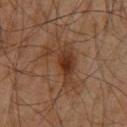Impression:
This lesion was catalogued during total-body skin photography and was not selected for biopsy.
Background:
From the chest. A 15 mm close-up extracted from a 3D total-body photography capture. A male subject, aged approximately 60. Automated tile analysis of the lesion measured an average lesion color of about L≈33 a*≈18 b*≈28 (CIELAB), a lesion–skin lightness drop of about 8, and a normalized lesion–skin contrast near 7.5. It also reported a border-irregularity index near 8.5/10.